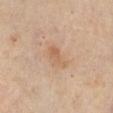Case summary:
• workup — imaged on a skin check; not biopsied